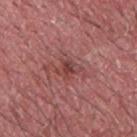Clinical impression:
Captured during whole-body skin photography for melanoma surveillance; the lesion was not biopsied.
Context:
A lesion tile, about 15 mm wide, cut from a 3D total-body photograph. From the chest. A male subject, about 40 years old.